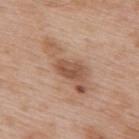The lesion was photographed on a routine skin check and not biopsied; there is no pathology result. From the upper back. Cropped from a whole-body photographic skin survey; the tile spans about 15 mm. The total-body-photography lesion software estimated a border-irregularity index near 7.5/10, a color-variation rating of about 4.5/10, and a peripheral color-asymmetry measure near 1.5. The analysis additionally found a nevus-likeness score of about 15/100. A male patient roughly 50 years of age. Approximately 7.5 mm at its widest. Imaged with white-light lighting.